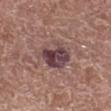Assessment: The lesion was photographed on a routine skin check and not biopsied; there is no pathology result. Background: The lesion is located on the right lower leg. This image is a 15 mm lesion crop taken from a total-body photograph. A male subject, about 80 years old.